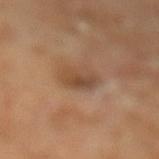notes = imaged on a skin check; not biopsied | image = ~15 mm crop, total-body skin-cancer survey | location = the left lower leg | patient = male, approximately 65 years of age | diameter = about 3 mm | automated lesion analysis = a mean CIELAB color near L≈46 a*≈19 b*≈31, roughly 9 lightness units darker than nearby skin, and a normalized lesion–skin contrast near 7; a border-irregularity rating of about 3/10 and a within-lesion color-variation index near 5.5/10; a classifier nevus-likeness of about 5/100 and a detector confidence of about 100 out of 100 that the crop contains a lesion.